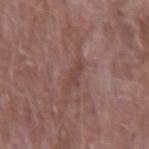workup = catalogued during a skin exam; not biopsied
lighting = white-light
body site = the right forearm
patient = male, roughly 65 years of age
imaging modality = 15 mm crop, total-body photography